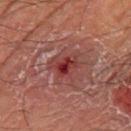The tile uses cross-polarized illumination. A male patient, about 55 years old. The lesion is located on the right thigh. The lesion's longest dimension is about 3.5 mm. The total-body-photography lesion software estimated an eccentricity of roughly 0.6 and a symmetry-axis asymmetry near 0.35. A lesion tile, about 15 mm wide, cut from a 3D total-body photograph.